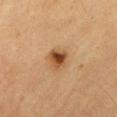Clinical impression:
Part of a total-body skin-imaging series; this lesion was reviewed on a skin check and was not flagged for biopsy.
Background:
Captured under cross-polarized illumination. A male subject, aged 83 or over. Located on the abdomen. A 15 mm close-up tile from a total-body photography series done for melanoma screening.